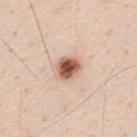  biopsy_status: not biopsied; imaged during a skin examination
  lesion_size:
    long_diameter_mm_approx: 3.0
  site: upper back
  image:
    source: total-body photography crop
    field_of_view_mm: 15
  patient:
    sex: male
    age_approx: 35